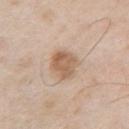follow-up=no biopsy performed (imaged during a skin exam)
tile lighting=white-light
size=~4 mm (longest diameter)
patient=male, roughly 55 years of age
location=the front of the torso
TBP lesion metrics=an automated nevus-likeness rating near 45 out of 100 and a lesion-detection confidence of about 100/100
acquisition=total-body-photography crop, ~15 mm field of view